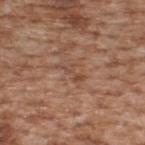| field | value |
|---|---|
| notes | catalogued during a skin exam; not biopsied |
| lesion diameter | ~3.5 mm (longest diameter) |
| TBP lesion metrics | a footprint of about 3.5 mm², a shape eccentricity near 0.9, and a shape-asymmetry score of about 0.55 (0 = symmetric); a classifier nevus-likeness of about 0/100 and a lesion-detection confidence of about 75/100 |
| image source | total-body-photography crop, ~15 mm field of view |
| subject | male, aged 63–67 |
| body site | the back |